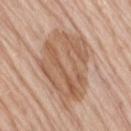Findings:
- follow-up — imaged on a skin check; not biopsied
- anatomic site — the right thigh
- lighting — white-light
- patient — female, in their mid-70s
- image — total-body-photography crop, ~15 mm field of view